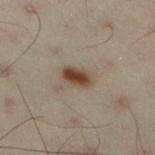Assessment: Captured during whole-body skin photography for melanoma surveillance; the lesion was not biopsied. Acquisition and patient details: Automated tile analysis of the lesion measured an area of roughly 5 mm² and a shape-asymmetry score of about 0.2 (0 = symmetric). The software also gave border irregularity of about 2 on a 0–10 scale, a color-variation rating of about 2.5/10, and radial color variation of about 1. About 3.5 mm across. This image is a 15 mm lesion crop taken from a total-body photograph. The lesion is located on the left leg. A male patient aged approximately 50. Imaged with cross-polarized lighting.